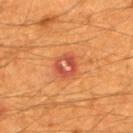No biopsy was performed on this lesion — it was imaged during a full skin examination and was not determined to be concerning. A region of skin cropped from a whole-body photographic capture, roughly 15 mm wide. The lesion's longest dimension is about 3 mm. The lesion-visualizer software estimated a mean CIELAB color near L≈50 a*≈32 b*≈36, a lesion–skin lightness drop of about 9, and a normalized border contrast of about 7.5. It also reported lesion-presence confidence of about 100/100. The lesion is located on the upper back. The patient is a male aged 58–62.